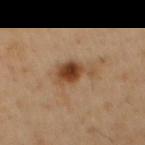Assessment:
Recorded during total-body skin imaging; not selected for excision or biopsy.
Acquisition and patient details:
The tile uses cross-polarized illumination. On the front of the torso. The recorded lesion diameter is about 4 mm. A 15 mm crop from a total-body photograph taken for skin-cancer surveillance. The subject is a male aged 48–52.